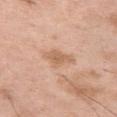The lesion was photographed on a routine skin check and not biopsied; there is no pathology result.
Approximately 3.5 mm at its widest.
A roughly 15 mm field-of-view crop from a total-body skin photograph.
Located on the left thigh.
A male patient, aged 48–52.
Imaged with white-light lighting.